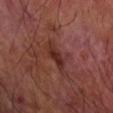<tbp_lesion>
<patient>
  <sex>male</sex>
  <age_approx>70</age_approx>
</patient>
<automated_metrics>
  <cielab_L>29</cielab_L>
  <cielab_a>24</cielab_a>
  <cielab_b>23</cielab_b>
  <vs_skin_darker_L>8.0</vs_skin_darker_L>
  <vs_skin_contrast_norm>8.0</vs_skin_contrast_norm>
  <color_variation_0_10>3.0</color_variation_0_10>
  <peripheral_color_asymmetry>1.0</peripheral_color_asymmetry>
</automated_metrics>
<site>right forearm</site>
<lesion_size>
  <long_diameter_mm_approx>3.5</long_diameter_mm_approx>
</lesion_size>
<image>
  <source>total-body photography crop</source>
  <field_of_view_mm>15</field_of_view_mm>
</image>
<lighting>cross-polarized</lighting>
</tbp_lesion>